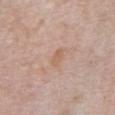Captured during whole-body skin photography for melanoma surveillance; the lesion was not biopsied.
Longest diameter approximately 2.5 mm.
The patient is a male aged around 60.
The lesion-visualizer software estimated two-axis asymmetry of about 0.2. It also reported border irregularity of about 2 on a 0–10 scale, a color-variation rating of about 1.5/10, and radial color variation of about 0.5.
Captured under white-light illumination.
A lesion tile, about 15 mm wide, cut from a 3D total-body photograph.
On the abdomen.